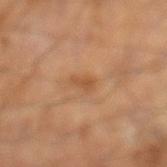{
  "patient": {
    "sex": "male",
    "age_approx": 65
  },
  "site": "left lower leg",
  "lesion_size": {
    "long_diameter_mm_approx": 3.0
  },
  "image": {
    "source": "total-body photography crop",
    "field_of_view_mm": 15
  },
  "automated_metrics": {
    "area_mm2_approx": 3.0,
    "shape_asymmetry": 0.45,
    "cielab_L": 51,
    "cielab_a": 21,
    "cielab_b": 36,
    "vs_skin_darker_L": 8.0,
    "vs_skin_contrast_norm": 6.0,
    "lesion_detection_confidence_0_100": 100
  }
}